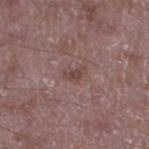| key | value |
|---|---|
| biopsy status | no biopsy performed (imaged during a skin exam) |
| image source | total-body-photography crop, ~15 mm field of view |
| site | the left lower leg |
| patient | male, aged approximately 50 |